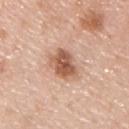Clinical impression:
Imaged during a routine full-body skin examination; the lesion was not biopsied and no histopathology is available.
Image and clinical context:
A roughly 15 mm field-of-view crop from a total-body skin photograph. The subject is a male approximately 45 years of age. On the chest.